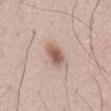| field | value |
|---|---|
| follow-up | no biopsy performed (imaged during a skin exam) |
| image | ~15 mm tile from a whole-body skin photo |
| body site | the leg |
| illumination | white-light |
| patient | male, aged 48 to 52 |
| lesion diameter | ~3 mm (longest diameter) |